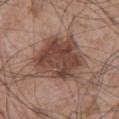This lesion was catalogued during total-body skin photography and was not selected for biopsy. The subject is a male approximately 70 years of age. Longest diameter approximately 6.5 mm. A 15 mm crop from a total-body photograph taken for skin-cancer surveillance. Automated image analysis of the tile measured a lesion area of about 28 mm², an eccentricity of roughly 0.4, and a symmetry-axis asymmetry near 0.25. And it measured an average lesion color of about L≈44 a*≈19 b*≈25 (CIELAB), a lesion–skin lightness drop of about 13, and a normalized lesion–skin contrast near 9.5. And it measured a classifier nevus-likeness of about 25/100 and a detector confidence of about 100 out of 100 that the crop contains a lesion. From the chest. This is a white-light tile.